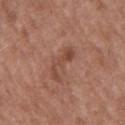Assessment: This lesion was catalogued during total-body skin photography and was not selected for biopsy. Image and clinical context: A 15 mm close-up tile from a total-body photography series done for melanoma screening. A male patient, aged 48–52. The lesion is located on the mid back. The recorded lesion diameter is about 4 mm. This is a white-light tile.